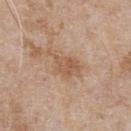Clinical impression: The lesion was photographed on a routine skin check and not biopsied; there is no pathology result. Background: Cropped from a whole-body photographic skin survey; the tile spans about 15 mm. The lesion is on the front of the torso. Longest diameter approximately 4.5 mm. The tile uses white-light illumination. The subject is a male aged 63–67.